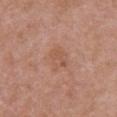No biopsy was performed on this lesion — it was imaged during a full skin examination and was not determined to be concerning.
Approximately 3 mm at its widest.
Located on the chest.
A female subject, in their 50s.
Cropped from a whole-body photographic skin survey; the tile spans about 15 mm.